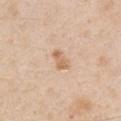Clinical impression:
Imaged during a routine full-body skin examination; the lesion was not biopsied and no histopathology is available.
Image and clinical context:
The lesion's longest dimension is about 2.5 mm. The lesion is located on the right upper arm. A male subject roughly 50 years of age. A roughly 15 mm field-of-view crop from a total-body skin photograph. Imaged with white-light lighting.